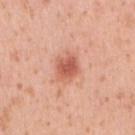notes: imaged on a skin check; not biopsied
image source: total-body-photography crop, ~15 mm field of view
size: ~3 mm (longest diameter)
location: the right upper arm
tile lighting: white-light illumination
patient: female, aged 38–42